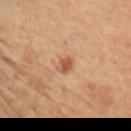Impression:
Recorded during total-body skin imaging; not selected for excision or biopsy.
Context:
A lesion tile, about 15 mm wide, cut from a 3D total-body photograph. About 2 mm across. Captured under cross-polarized illumination. The total-body-photography lesion software estimated a mean CIELAB color near L≈53 a*≈22 b*≈36 and roughly 10 lightness units darker than nearby skin. The analysis additionally found internal color variation of about 2 on a 0–10 scale. From the left upper arm. The subject is a female aged 68–72.